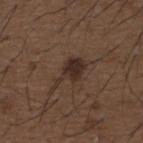Q: Was a biopsy performed?
A: total-body-photography surveillance lesion; no biopsy
Q: Illumination type?
A: white-light illumination
Q: Lesion location?
A: the upper back
Q: How was this image acquired?
A: total-body-photography crop, ~15 mm field of view
Q: Who is the patient?
A: male, about 50 years old